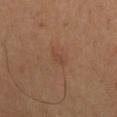Clinical impression: Captured during whole-body skin photography for melanoma surveillance; the lesion was not biopsied. Background: The lesion-visualizer software estimated a footprint of about 2 mm², an outline eccentricity of about 0.8 (0 = round, 1 = elongated), and two-axis asymmetry of about 0.35. It also reported internal color variation of about 0 on a 0–10 scale. The analysis additionally found lesion-presence confidence of about 100/100. Approximately 2 mm at its widest. A male subject in their mid-60s. A 15 mm close-up tile from a total-body photography series done for melanoma screening. On the left upper arm. The tile uses cross-polarized illumination.